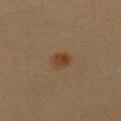{
  "biopsy_status": "not biopsied; imaged during a skin examination",
  "site": "left upper arm",
  "automated_metrics": {
    "border_irregularity_0_10": 2.5,
    "color_variation_0_10": 3.0,
    "peripheral_color_asymmetry": 1.0,
    "nevus_likeness_0_100": 95,
    "lesion_detection_confidence_0_100": 100
  },
  "image": {
    "source": "total-body photography crop",
    "field_of_view_mm": 15
  },
  "patient": {
    "sex": "female",
    "age_approx": 20
  }
}